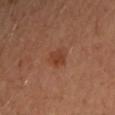follow-up: imaged on a skin check; not biopsied
imaging modality: ~15 mm crop, total-body skin-cancer survey
subject: female, roughly 40 years of age
automated lesion analysis: an area of roughly 4.5 mm² and a shape-asymmetry score of about 0.25 (0 = symmetric); a border-irregularity index near 2.5/10, internal color variation of about 2 on a 0–10 scale, and peripheral color asymmetry of about 0.5; an automated nevus-likeness rating near 50 out of 100 and lesion-presence confidence of about 100/100
body site: the left forearm
size: ~2.5 mm (longest diameter)